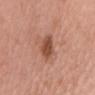The lesion was tiled from a total-body skin photograph and was not biopsied. The subject is a female in their 50s. Longest diameter approximately 3 mm. Automated image analysis of the tile measured an automated nevus-likeness rating near 80 out of 100 and lesion-presence confidence of about 100/100. A close-up tile cropped from a whole-body skin photograph, about 15 mm across. Captured under white-light illumination. The lesion is on the head or neck.